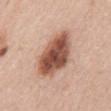Assessment: The lesion was tiled from a total-body skin photograph and was not biopsied. Clinical summary: The total-body-photography lesion software estimated a lesion area of about 20 mm², a shape eccentricity near 0.7, and two-axis asymmetry of about 0.15. A region of skin cropped from a whole-body photographic capture, roughly 15 mm wide. The lesion's longest dimension is about 6 mm. A female patient, in their 40s. The lesion is on the mid back.